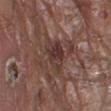Findings:
• site — the left lower leg
• lighting — white-light
• subject — male, in their 80s
• imaging modality — ~15 mm tile from a whole-body skin photo